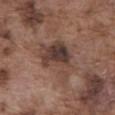{"biopsy_status": "not biopsied; imaged during a skin examination", "image": {"source": "total-body photography crop", "field_of_view_mm": 15}, "lighting": "white-light", "automated_metrics": {"nevus_likeness_0_100": 0, "lesion_detection_confidence_0_100": 100}, "lesion_size": {"long_diameter_mm_approx": 5.5}, "site": "abdomen", "patient": {"sex": "male", "age_approx": 75}}